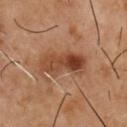workup: total-body-photography surveillance lesion; no biopsy
imaging modality: ~15 mm tile from a whole-body skin photo
site: the chest
subject: male, approximately 55 years of age